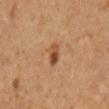notes: imaged on a skin check; not biopsied | patient: male, aged around 60 | size: ≈3 mm | automated lesion analysis: a footprint of about 4.5 mm², an eccentricity of roughly 0.85, and a symmetry-axis asymmetry near 0.3; about 10 CIELAB-L* units darker than the surrounding skin and a normalized lesion–skin contrast near 8; a border-irregularity index near 3/10, internal color variation of about 7.5 on a 0–10 scale, and radial color variation of about 3 | image source: total-body-photography crop, ~15 mm field of view | anatomic site: the mid back.